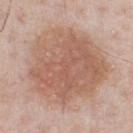workup — catalogued during a skin exam; not biopsied | automated lesion analysis — a within-lesion color-variation index near 4.5/10 and radial color variation of about 1.5; an automated nevus-likeness rating near 35 out of 100 and a lesion-detection confidence of about 100/100 | patient — male, approximately 60 years of age | imaging modality — ~15 mm crop, total-body skin-cancer survey | lesion diameter — ≈9 mm | illumination — white-light | anatomic site — the front of the torso.